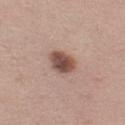Impression:
The lesion was tiled from a total-body skin photograph and was not biopsied.
Context:
The total-body-photography lesion software estimated a classifier nevus-likeness of about 85/100 and a detector confidence of about 100 out of 100 that the crop contains a lesion. A close-up tile cropped from a whole-body skin photograph, about 15 mm across. The lesion is located on the left thigh. A female subject in their mid- to late 40s.